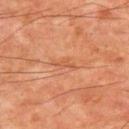Case summary:
* biopsy status: catalogued during a skin exam; not biopsied
* illumination: cross-polarized illumination
* subject: male, in their mid- to late 60s
* image-analysis metrics: a classifier nevus-likeness of about 0/100 and a detector confidence of about 100 out of 100 that the crop contains a lesion
* acquisition: 15 mm crop, total-body photography
* lesion size: about 3 mm
* location: the upper back